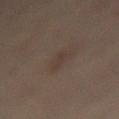Q: Is there a histopathology result?
A: total-body-photography surveillance lesion; no biopsy
Q: How was the tile lit?
A: cross-polarized
Q: How large is the lesion?
A: ~3 mm (longest diameter)
Q: Where on the body is the lesion?
A: the back
Q: What kind of image is this?
A: 15 mm crop, total-body photography
Q: Automated lesion metrics?
A: an area of roughly 3 mm², an eccentricity of roughly 0.9, and a symmetry-axis asymmetry near 0.35; a classifier nevus-likeness of about 5/100
Q: Who is the patient?
A: female, in their mid- to late 50s